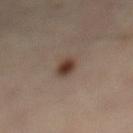workup = no biopsy performed (imaged during a skin exam)
body site = the mid back
size = about 3 mm
tile lighting = cross-polarized illumination
acquisition = ~15 mm tile from a whole-body skin photo
subject = aged 53–57
automated lesion analysis = a lesion color around L≈39 a*≈15 b*≈24 in CIELAB and about 12 CIELAB-L* units darker than the surrounding skin; lesion-presence confidence of about 100/100